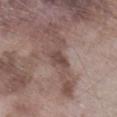Q: Was this lesion biopsied?
A: total-body-photography surveillance lesion; no biopsy
Q: How was this image acquired?
A: ~15 mm tile from a whole-body skin photo
Q: What are the patient's age and sex?
A: male, aged 58 to 62
Q: Lesion location?
A: the leg
Q: How large is the lesion?
A: about 3 mm